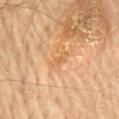Clinical impression: Recorded during total-body skin imaging; not selected for excision or biopsy. Context: The patient is a male about 65 years old. On the chest. This image is a 15 mm lesion crop taken from a total-body photograph. The lesion's longest dimension is about 3 mm. Imaged with cross-polarized lighting.